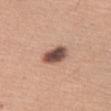Q: Was this lesion biopsied?
A: imaged on a skin check; not biopsied
Q: What is the imaging modality?
A: 15 mm crop, total-body photography
Q: What are the patient's age and sex?
A: female, in their mid- to late 50s
Q: How was the tile lit?
A: white-light
Q: Automated lesion metrics?
A: a lesion area of about 7 mm², an outline eccentricity of about 0.7 (0 = round, 1 = elongated), and two-axis asymmetry of about 0.15; a mean CIELAB color near L≈50 a*≈19 b*≈25, about 18 CIELAB-L* units darker than the surrounding skin, and a normalized lesion–skin contrast near 12
Q: Lesion size?
A: ≈3.5 mm
Q: Lesion location?
A: the right thigh